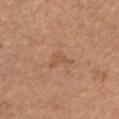Q: Patient demographics?
A: female, aged 48 to 52
Q: Lesion location?
A: the chest
Q: How was this image acquired?
A: ~15 mm crop, total-body skin-cancer survey